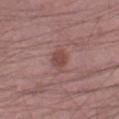Notes:
• follow-up: catalogued during a skin exam; not biopsied
• automated metrics: a nevus-likeness score of about 70/100 and lesion-presence confidence of about 100/100
• acquisition: ~15 mm tile from a whole-body skin photo
• lesion size: ≈2.5 mm
• tile lighting: white-light illumination
• anatomic site: the right thigh
• subject: male, in their mid-50s